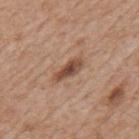Clinical impression: Recorded during total-body skin imaging; not selected for excision or biopsy. Clinical summary: A male patient, aged approximately 65. The recorded lesion diameter is about 4 mm. A lesion tile, about 15 mm wide, cut from a 3D total-body photograph. Located on the back. Captured under white-light illumination.